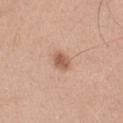{
  "biopsy_status": "not biopsied; imaged during a skin examination",
  "image": {
    "source": "total-body photography crop",
    "field_of_view_mm": 15
  },
  "patient": {
    "sex": "male",
    "age_approx": 30
  },
  "site": "front of the torso",
  "lesion_size": {
    "long_diameter_mm_approx": 2.5
  }
}